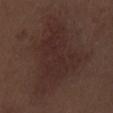Q: Was this lesion biopsied?
A: total-body-photography surveillance lesion; no biopsy
Q: What kind of image is this?
A: 15 mm crop, total-body photography
Q: Patient demographics?
A: male, approximately 70 years of age
Q: How large is the lesion?
A: ≈11.5 mm
Q: Lesion location?
A: the left thigh